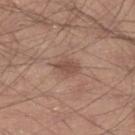This lesion was catalogued during total-body skin photography and was not selected for biopsy. A male patient in their 30s. A region of skin cropped from a whole-body photographic capture, roughly 15 mm wide. Located on the right lower leg. An algorithmic analysis of the crop reported a mean CIELAB color near L≈49 a*≈19 b*≈26. This is a white-light tile.